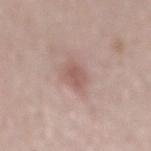<record>
  <biopsy_status>not biopsied; imaged during a skin examination</biopsy_status>
  <image>
    <source>total-body photography crop</source>
    <field_of_view_mm>15</field_of_view_mm>
  </image>
  <site>mid back</site>
  <patient>
    <sex>male</sex>
    <age_approx>55</age_approx>
  </patient>
</record>